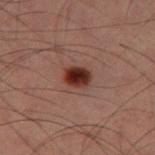Impression:
Imaged during a routine full-body skin examination; the lesion was not biopsied and no histopathology is available.
Clinical summary:
A male subject aged approximately 60. A 15 mm crop from a total-body photograph taken for skin-cancer surveillance. Located on the left thigh. Captured under cross-polarized illumination. Automated image analysis of the tile measured border irregularity of about 1.5 on a 0–10 scale, a color-variation rating of about 5/10, and a peripheral color-asymmetry measure near 1.5.